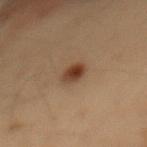Impression:
Imaged during a routine full-body skin examination; the lesion was not biopsied and no histopathology is available.
Clinical summary:
Cropped from a whole-body photographic skin survey; the tile spans about 15 mm. An algorithmic analysis of the crop reported a lesion color around L≈32 a*≈16 b*≈25 in CIELAB, a lesion–skin lightness drop of about 11, and a normalized border contrast of about 10. The analysis additionally found an automated nevus-likeness rating near 100 out of 100. The lesion is located on the mid back. The patient is a male roughly 55 years of age. About 2.5 mm across. This is a cross-polarized tile.